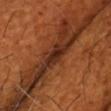The lesion was photographed on a routine skin check and not biopsied; there is no pathology result. Approximately 3 mm at its widest. A close-up tile cropped from a whole-body skin photograph, about 15 mm across. The lesion is on the head or neck. Automated image analysis of the tile measured an automated nevus-likeness rating near 0 out of 100. A male subject approximately 65 years of age.